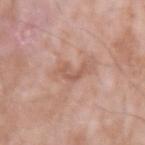The lesion was tiled from a total-body skin photograph and was not biopsied. A roughly 15 mm field-of-view crop from a total-body skin photograph. Longest diameter approximately 3.5 mm. From the left upper arm. A male subject, roughly 75 years of age. Captured under white-light illumination.